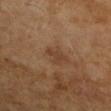{"site": "right upper arm", "image": {"source": "total-body photography crop", "field_of_view_mm": 15}, "patient": {"sex": "female", "age_approx": 60}}